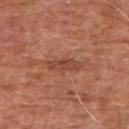The lesion was tiled from a total-body skin photograph and was not biopsied. A male subject, in their mid- to late 70s. From the right upper arm. A lesion tile, about 15 mm wide, cut from a 3D total-body photograph.